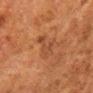  biopsy_status: not biopsied; imaged during a skin examination
  automated_metrics:
    cielab_L: 36
    cielab_a: 20
    cielab_b: 29
    vs_skin_darker_L: 6.0
  lesion_size:
    long_diameter_mm_approx: 4.5
  patient:
    sex: male
    age_approx: 80
  site: leg
  image:
    source: total-body photography crop
    field_of_view_mm: 15